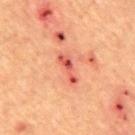image: ~15 mm crop, total-body skin-cancer survey; anatomic site: the back; tile lighting: cross-polarized; patient: male, in their mid- to late 50s.